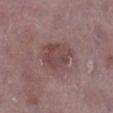follow-up: imaged on a skin check; not biopsied | subject: male, aged around 75 | imaging modality: 15 mm crop, total-body photography | illumination: white-light | diameter: ≈4.5 mm | site: the leg.